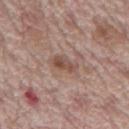The lesion was tiled from a total-body skin photograph and was not biopsied.
A lesion tile, about 15 mm wide, cut from a 3D total-body photograph.
Measured at roughly 2.5 mm in maximum diameter.
On the mid back.
Captured under white-light illumination.
The patient is a male in their 70s.